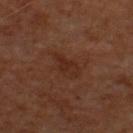biopsy status: catalogued during a skin exam; not biopsied
image-analysis metrics: an area of roughly 6 mm², a shape eccentricity near 0.85, and a symmetry-axis asymmetry near 0.35; a lesion color around L≈25 a*≈19 b*≈24 in CIELAB, a lesion–skin lightness drop of about 5, and a normalized border contrast of about 5.5; a border-irregularity rating of about 3.5/10, internal color variation of about 2 on a 0–10 scale, and radial color variation of about 0.5; a nevus-likeness score of about 0/100 and lesion-presence confidence of about 100/100
acquisition: ~15 mm crop, total-body skin-cancer survey
patient: male, aged approximately 60
diameter: ≈3.5 mm
site: the upper back
tile lighting: cross-polarized illumination